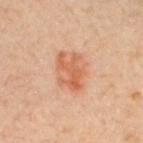Q: Lesion size?
A: ~5 mm (longest diameter)
Q: What kind of image is this?
A: total-body-photography crop, ~15 mm field of view
Q: Who is the patient?
A: female, aged approximately 30
Q: Automated lesion metrics?
A: a normalized border contrast of about 6.5; an automated nevus-likeness rating near 60 out of 100 and lesion-presence confidence of about 100/100
Q: Where on the body is the lesion?
A: the chest